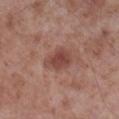Measured at roughly 4 mm in maximum diameter.
Imaged with white-light lighting.
A 15 mm close-up extracted from a 3D total-body photography capture.
A male patient aged 53 to 57.
Located on the leg.